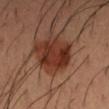Notes:
• workup: imaged on a skin check; not biopsied
• tile lighting: cross-polarized illumination
• image source: 15 mm crop, total-body photography
• patient: male, in their 40s
• automated lesion analysis: an average lesion color of about L≈32 a*≈24 b*≈28 (CIELAB) and roughly 12 lightness units darker than nearby skin
• site: the arm
• lesion diameter: ~6 mm (longest diameter)